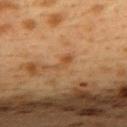Recorded during total-body skin imaging; not selected for excision or biopsy.
Imaged with cross-polarized lighting.
A female subject about 40 years old.
The lesion-visualizer software estimated a border-irregularity index near 3.5/10, internal color variation of about 1 on a 0–10 scale, and peripheral color asymmetry of about 0. It also reported a classifier nevus-likeness of about 0/100 and lesion-presence confidence of about 100/100.
From the upper back.
Approximately 3 mm at its widest.
A roughly 15 mm field-of-view crop from a total-body skin photograph.